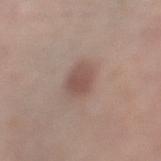workup: imaged on a skin check; not biopsied
anatomic site: the left thigh
subject: female, roughly 40 years of age
TBP lesion metrics: a mean CIELAB color near L≈51 a*≈18 b*≈23, about 9 CIELAB-L* units darker than the surrounding skin, and a normalized lesion–skin contrast near 7
diameter: ~3 mm (longest diameter)
illumination: white-light illumination
acquisition: ~15 mm tile from a whole-body skin photo